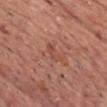The lesion is on the front of the torso.
A male patient aged around 65.
A close-up tile cropped from a whole-body skin photograph, about 15 mm across.
Automated image analysis of the tile measured an area of roughly 3 mm², an outline eccentricity of about 0.85 (0 = round, 1 = elongated), and a symmetry-axis asymmetry near 0.4. The software also gave a lesion color around L≈49 a*≈26 b*≈30 in CIELAB and a lesion-to-skin contrast of about 5 (normalized; higher = more distinct). And it measured a border-irregularity index near 5.5/10, a color-variation rating of about 0/10, and a peripheral color-asymmetry measure near 0. And it measured a classifier nevus-likeness of about 0/100 and a lesion-detection confidence of about 100/100.
Captured under white-light illumination.